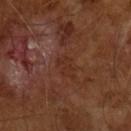Q: Was this lesion biopsied?
A: imaged on a skin check; not biopsied
Q: Who is the patient?
A: male, roughly 65 years of age
Q: How was this image acquired?
A: ~15 mm tile from a whole-body skin photo